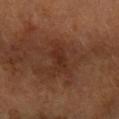Q: What lighting was used for the tile?
A: cross-polarized
Q: What is the lesion's diameter?
A: ≈3.5 mm
Q: What kind of image is this?
A: ~15 mm tile from a whole-body skin photo
Q: Lesion location?
A: the right forearm
Q: What are the patient's age and sex?
A: female
Q: What did automated image analysis measure?
A: an average lesion color of about L≈30 a*≈22 b*≈28 (CIELAB) and a lesion-to-skin contrast of about 6 (normalized; higher = more distinct); border irregularity of about 4 on a 0–10 scale, internal color variation of about 1.5 on a 0–10 scale, and a peripheral color-asymmetry measure near 0.5; an automated nevus-likeness rating near 0 out of 100 and lesion-presence confidence of about 100/100